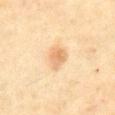{"biopsy_status": "not biopsied; imaged during a skin examination", "site": "abdomen", "lesion_size": {"long_diameter_mm_approx": 3.0}, "image": {"source": "total-body photography crop", "field_of_view_mm": 15}, "lighting": "cross-polarized", "patient": {"sex": "male", "age_approx": 70}}